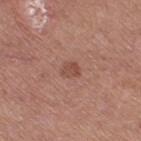Q: Lesion location?
A: the right thigh
Q: What are the patient's age and sex?
A: female, aged around 30
Q: What is the imaging modality?
A: 15 mm crop, total-body photography
Q: What is the histopathologic diagnosis?
A: a verruca